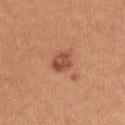The lesion was tiled from a total-body skin photograph and was not biopsied. A female patient aged 23 to 27. Cropped from a total-body skin-imaging series; the visible field is about 15 mm. The lesion-visualizer software estimated an area of roughly 4.5 mm², an outline eccentricity of about 0.6 (0 = round, 1 = elongated), and two-axis asymmetry of about 0.2. From the left upper arm. Approximately 2.5 mm at its widest.